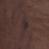{"biopsy_status": "not biopsied; imaged during a skin examination", "site": "left thigh", "image": {"source": "total-body photography crop", "field_of_view_mm": 15}, "patient": {"sex": "male", "age_approx": 75}}